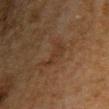Case summary:
• follow-up · catalogued during a skin exam; not biopsied
• subject · male, aged 48 to 52
• size · ≈3 mm
• image-analysis metrics · a lesion area of about 4 mm²; a lesion color around L≈27 a*≈15 b*≈25 in CIELAB, roughly 4 lightness units darker than nearby skin, and a normalized lesion–skin contrast near 5; an automated nevus-likeness rating near 0 out of 100 and lesion-presence confidence of about 100/100
• illumination · cross-polarized
• site · the chest
• image source · ~15 mm crop, total-body skin-cancer survey